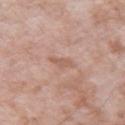Image and clinical context:
A male patient, aged 73 to 77. The lesion is located on the left upper arm. The recorded lesion diameter is about 3 mm. A close-up tile cropped from a whole-body skin photograph, about 15 mm across. This is a white-light tile.